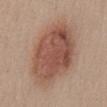Assessment: No biopsy was performed on this lesion — it was imaged during a full skin examination and was not determined to be concerning. Clinical summary: Approximately 8.5 mm at its widest. A 15 mm close-up tile from a total-body photography series done for melanoma screening. A female patient, in their mid-40s. The tile uses white-light illumination. Located on the abdomen. The lesion-visualizer software estimated a lesion color around L≈51 a*≈21 b*≈27 in CIELAB, roughly 12 lightness units darker than nearby skin, and a lesion-to-skin contrast of about 8.5 (normalized; higher = more distinct). The software also gave a border-irregularity index near 1.5/10, a within-lesion color-variation index near 5.5/10, and peripheral color asymmetry of about 2. The software also gave a nevus-likeness score of about 100/100 and a detector confidence of about 100 out of 100 that the crop contains a lesion.